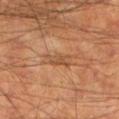Q: Was a biopsy performed?
A: no biopsy performed (imaged during a skin exam)